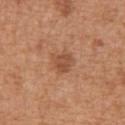Clinical impression: No biopsy was performed on this lesion — it was imaged during a full skin examination and was not determined to be concerning. Clinical summary: The tile uses white-light illumination. Measured at roughly 3 mm in maximum diameter. The lesion is on the abdomen. The lesion-visualizer software estimated an area of roughly 6 mm² and two-axis asymmetry of about 0.25. This image is a 15 mm lesion crop taken from a total-body photograph. The patient is a male in their mid- to late 60s.